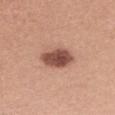{"biopsy_status": "not biopsied; imaged during a skin examination", "automated_metrics": {"cielab_L": 51, "cielab_a": 23, "cielab_b": 27, "vs_skin_darker_L": 16.0, "vs_skin_contrast_norm": 10.5, "border_irregularity_0_10": 2.0, "peripheral_color_asymmetry": 2.0}, "image": {"source": "total-body photography crop", "field_of_view_mm": 15}, "patient": {"sex": "female", "age_approx": 35}, "site": "upper back", "lighting": "white-light", "lesion_size": {"long_diameter_mm_approx": 4.5}}